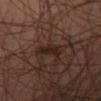Impression: Part of a total-body skin-imaging series; this lesion was reviewed on a skin check and was not flagged for biopsy. Acquisition and patient details: A male subject, aged 43–47. The lesion is located on the right thigh. This image is a 15 mm lesion crop taken from a total-body photograph.